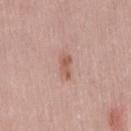Clinical summary:
A female subject aged approximately 40. On the lower back. The total-body-photography lesion software estimated an area of roughly 3.5 mm², an eccentricity of roughly 0.85, and a shape-asymmetry score of about 0.3 (0 = symmetric). The software also gave a within-lesion color-variation index near 2/10 and radial color variation of about 0.5. The software also gave an automated nevus-likeness rating near 0 out of 100. Measured at roughly 2.5 mm in maximum diameter. Cropped from a total-body skin-imaging series; the visible field is about 15 mm.